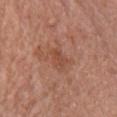<case>
<biopsy_status>not biopsied; imaged during a skin examination</biopsy_status>
<lesion_size>
  <long_diameter_mm_approx>2.5</long_diameter_mm_approx>
</lesion_size>
<lighting>white-light</lighting>
<patient>
  <sex>female</sex>
  <age_approx>65</age_approx>
</patient>
<site>chest</site>
<image>
  <source>total-body photography crop</source>
  <field_of_view_mm>15</field_of_view_mm>
</image>
</case>